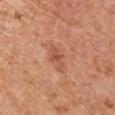Part of a total-body skin-imaging series; this lesion was reviewed on a skin check and was not flagged for biopsy.
Longest diameter approximately 3.5 mm.
This image is a 15 mm lesion crop taken from a total-body photograph.
A male patient aged 63–67.
The total-body-photography lesion software estimated a shape eccentricity near 0.9 and a symmetry-axis asymmetry near 0.4.
Located on the left upper arm.
Imaged with white-light lighting.